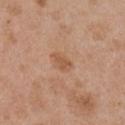Part of a total-body skin-imaging series; this lesion was reviewed on a skin check and was not flagged for biopsy. A female subject, roughly 40 years of age. Cropped from a total-body skin-imaging series; the visible field is about 15 mm. The tile uses white-light illumination. The lesion-visualizer software estimated a footprint of about 4 mm², an eccentricity of roughly 0.8, and a shape-asymmetry score of about 0.3 (0 = symmetric). And it measured a within-lesion color-variation index near 2/10 and a peripheral color-asymmetry measure near 1. It also reported a detector confidence of about 100 out of 100 that the crop contains a lesion. The recorded lesion diameter is about 3 mm. Located on the chest.